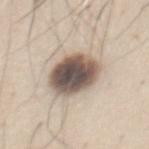Clinical impression:
Part of a total-body skin-imaging series; this lesion was reviewed on a skin check and was not flagged for biopsy.
Context:
Imaged with white-light lighting. Longest diameter approximately 5.5 mm. A male patient aged around 45. A 15 mm close-up extracted from a 3D total-body photography capture. Located on the abdomen.